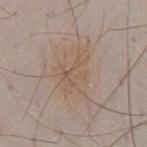Q: Was a biopsy performed?
A: total-body-photography surveillance lesion; no biopsy
Q: Patient demographics?
A: male, aged approximately 50
Q: What is the anatomic site?
A: the abdomen
Q: How was this image acquired?
A: ~15 mm tile from a whole-body skin photo
Q: Automated lesion metrics?
A: a mean CIELAB color near L≈55 a*≈14 b*≈27 and a lesion-to-skin contrast of about 5 (normalized; higher = more distinct); a border-irregularity rating of about 6.5/10 and a color-variation rating of about 2.5/10; lesion-presence confidence of about 100/100
Q: Illumination type?
A: white-light
Q: How large is the lesion?
A: ≈5 mm